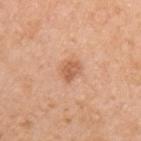Imaged during a routine full-body skin examination; the lesion was not biopsied and no histopathology is available. Cropped from a whole-body photographic skin survey; the tile spans about 15 mm. The lesion is on the left upper arm. Approximately 2.5 mm at its widest. Captured under white-light illumination. A female patient about 55 years old. Automated tile analysis of the lesion measured a shape eccentricity near 0.55 and a symmetry-axis asymmetry near 0.25. The software also gave about 10 CIELAB-L* units darker than the surrounding skin and a normalized lesion–skin contrast near 6.5. And it measured border irregularity of about 2.5 on a 0–10 scale, a color-variation rating of about 2.5/10, and a peripheral color-asymmetry measure near 1.